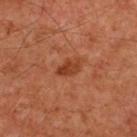follow-up: imaged on a skin check; not biopsied
patient: male, aged 58 to 62
image: total-body-photography crop, ~15 mm field of view
TBP lesion metrics: a lesion area of about 4 mm², an eccentricity of roughly 0.8, and two-axis asymmetry of about 0.25; an automated nevus-likeness rating near 35 out of 100 and lesion-presence confidence of about 100/100
body site: the chest
diameter: about 2.5 mm